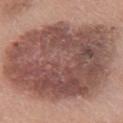This lesion was catalogued during total-body skin photography and was not selected for biopsy. The lesion is on the chest. A roughly 15 mm field-of-view crop from a total-body skin photograph. The subject is a female aged approximately 60.